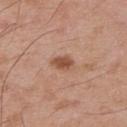The lesion was photographed on a routine skin check and not biopsied; there is no pathology result. A 15 mm close-up extracted from a 3D total-body photography capture. The lesion is on the upper back. An algorithmic analysis of the crop reported a footprint of about 4.5 mm², an outline eccentricity of about 0.75 (0 = round, 1 = elongated), and two-axis asymmetry of about 0.25. The analysis additionally found an average lesion color of about L≈52 a*≈22 b*≈32 (CIELAB), a lesion–skin lightness drop of about 11, and a lesion-to-skin contrast of about 8 (normalized; higher = more distinct). The software also gave lesion-presence confidence of about 100/100. A male patient, in their mid- to late 50s.